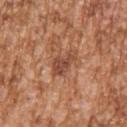Findings:
• follow-up — imaged on a skin check; not biopsied
• patient — male, roughly 45 years of age
• acquisition — ~15 mm crop, total-body skin-cancer survey
• location — the right upper arm